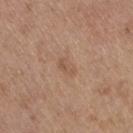Part of a total-body skin-imaging series; this lesion was reviewed on a skin check and was not flagged for biopsy.
This image is a 15 mm lesion crop taken from a total-body photograph.
The lesion is located on the right thigh.
A female subject, approximately 65 years of age.
Automated image analysis of the tile measured a lesion color around L≈54 a*≈18 b*≈31 in CIELAB.
Approximately 2.5 mm at its widest.
Imaged with white-light lighting.